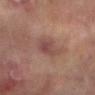biopsy status: total-body-photography surveillance lesion; no biopsy | lesion diameter: ≈4 mm | illumination: cross-polarized illumination | patient: female, aged 78–82 | image-analysis metrics: a classifier nevus-likeness of about 10/100 | site: the right arm | imaging modality: ~15 mm tile from a whole-body skin photo.